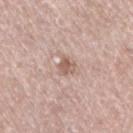biopsy status = catalogued during a skin exam; not biopsied
imaging modality = ~15 mm tile from a whole-body skin photo
body site = the right thigh
lighting = white-light
patient = female, aged 58–62
lesion size = ~2.5 mm (longest diameter)